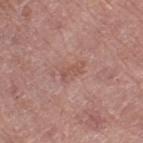biopsy_status: not biopsied; imaged during a skin examination
automated_metrics:
  border_irregularity_0_10: 3.5
  color_variation_0_10: 2.0
  peripheral_color_asymmetry: 0.5
patient:
  sex: female
  age_approx: 80
image:
  source: total-body photography crop
  field_of_view_mm: 15
site: left thigh